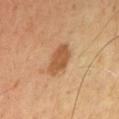{
  "biopsy_status": "not biopsied; imaged during a skin examination",
  "automated_metrics": {
    "area_mm2_approx": 8.0,
    "eccentricity": 0.75,
    "shape_asymmetry": 0.2,
    "cielab_L": 53,
    "cielab_a": 21,
    "cielab_b": 37,
    "vs_skin_darker_L": 11.0,
    "vs_skin_contrast_norm": 8.0,
    "nevus_likeness_0_100": 75,
    "lesion_detection_confidence_0_100": 100
  },
  "lighting": "cross-polarized",
  "lesion_size": {
    "long_diameter_mm_approx": 4.0
  },
  "image": {
    "source": "total-body photography crop",
    "field_of_view_mm": 15
  },
  "patient": {
    "sex": "male",
    "age_approx": 65
  }
}